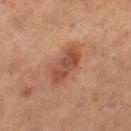Findings:
– notes · no biopsy performed (imaged during a skin exam)
– imaging modality · total-body-photography crop, ~15 mm field of view
– lighting · cross-polarized illumination
– site · the left thigh
– subject · female, in their mid- to late 50s
– lesion size · ~5 mm (longest diameter)